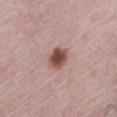size = ~3 mm (longest diameter)
anatomic site = the leg
patient = female, roughly 65 years of age
automated lesion analysis = a lesion color around L≈50 a*≈22 b*≈23 in CIELAB, about 15 CIELAB-L* units darker than the surrounding skin, and a normalized border contrast of about 10.5; a border-irregularity rating of about 1.5/10 and a color-variation rating of about 4/10; lesion-presence confidence of about 100/100
illumination = white-light
imaging modality = ~15 mm crop, total-body skin-cancer survey